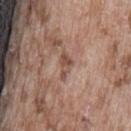Impression:
The lesion was photographed on a routine skin check and not biopsied; there is no pathology result.
Image and clinical context:
Cropped from a total-body skin-imaging series; the visible field is about 15 mm. Imaged with white-light lighting. A male subject, aged around 75. On the lower back. Approximately 3 mm at its widest.